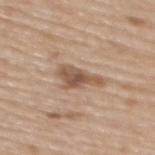workup = total-body-photography surveillance lesion; no biopsy | acquisition = total-body-photography crop, ~15 mm field of view | location = the upper back | diameter = about 4.5 mm | subject = female, aged approximately 70 | automated metrics = a classifier nevus-likeness of about 20/100 and a detector confidence of about 100 out of 100 that the crop contains a lesion | lighting = white-light illumination.